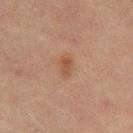workup — no biopsy performed (imaged during a skin exam)
location — the back
lighting — cross-polarized illumination
automated metrics — a footprint of about 3 mm² and two-axis asymmetry of about 0.3; an automated nevus-likeness rating near 75 out of 100 and a lesion-detection confidence of about 100/100
acquisition — total-body-photography crop, ~15 mm field of view
lesion diameter — ~2.5 mm (longest diameter)
subject — male, roughly 65 years of age